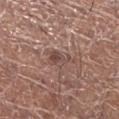site = the left lower leg | image = total-body-photography crop, ~15 mm field of view | patient = female, aged around 50 | illumination = white-light illumination | diameter = about 3.5 mm | TBP lesion metrics = an average lesion color of about L≈47 a*≈18 b*≈22 (CIELAB), a lesion–skin lightness drop of about 8, and a normalized border contrast of about 6.5.